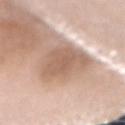Assessment: The lesion was photographed on a routine skin check and not biopsied; there is no pathology result. Background: The patient is a female roughly 60 years of age. Measured at roughly 6.5 mm in maximum diameter. From the mid back. Automated image analysis of the tile measured a footprint of about 18 mm², an eccentricity of roughly 0.8, and a shape-asymmetry score of about 0.25 (0 = symmetric). The software also gave border irregularity of about 3 on a 0–10 scale and peripheral color asymmetry of about 0.5. The analysis additionally found a nevus-likeness score of about 0/100 and a lesion-detection confidence of about 100/100. A 15 mm crop from a total-body photograph taken for skin-cancer surveillance.